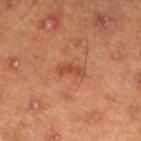Imaged during a routine full-body skin examination; the lesion was not biopsied and no histopathology is available. Cropped from a whole-body photographic skin survey; the tile spans about 15 mm. Captured under cross-polarized illumination. A male patient, about 45 years old. The lesion-visualizer software estimated an eccentricity of roughly 0.9. The lesion is on the left lower leg.